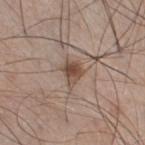Impression:
Part of a total-body skin-imaging series; this lesion was reviewed on a skin check and was not flagged for biopsy.
Context:
The lesion-visualizer software estimated roughly 12 lightness units darker than nearby skin and a normalized border contrast of about 9. The software also gave border irregularity of about 3.5 on a 0–10 scale, a color-variation rating of about 4.5/10, and a peripheral color-asymmetry measure near 2. And it measured a nevus-likeness score of about 85/100 and lesion-presence confidence of about 100/100. A male patient, roughly 60 years of age. The tile uses white-light illumination. Longest diameter approximately 3 mm. A lesion tile, about 15 mm wide, cut from a 3D total-body photograph. The lesion is located on the right thigh.